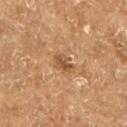biopsy status: total-body-photography surveillance lesion; no biopsy
lesion diameter: ~3 mm (longest diameter)
patient: male, in their mid-70s
image-analysis metrics: an outline eccentricity of about 0.75 (0 = round, 1 = elongated) and a symmetry-axis asymmetry near 0.25; a lesion color around L≈40 a*≈18 b*≈29 in CIELAB, roughly 9 lightness units darker than nearby skin, and a normalized lesion–skin contrast near 7.5; a border-irregularity index near 3/10; an automated nevus-likeness rating near 5 out of 100 and a detector confidence of about 100 out of 100 that the crop contains a lesion
image: 15 mm crop, total-body photography
body site: the left lower leg